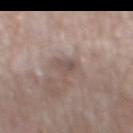Recorded during total-body skin imaging; not selected for excision or biopsy. A roughly 15 mm field-of-view crop from a total-body skin photograph. A male subject, aged 63–67. The lesion's longest dimension is about 2.5 mm. The tile uses white-light illumination. On the mid back.